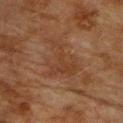Assessment: No biopsy was performed on this lesion — it was imaged during a full skin examination and was not determined to be concerning. Image and clinical context: An algorithmic analysis of the crop reported a lesion area of about 14 mm², an eccentricity of roughly 0.65, and a shape-asymmetry score of about 0.6 (0 = symmetric). It also reported a border-irregularity rating of about 7.5/10 and peripheral color asymmetry of about 1. It also reported a nevus-likeness score of about 0/100 and a detector confidence of about 100 out of 100 that the crop contains a lesion. A close-up tile cropped from a whole-body skin photograph, about 15 mm across. Captured under cross-polarized illumination. A male patient aged approximately 75. On the chest.